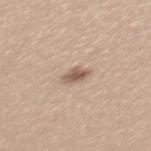Case summary:
– biopsy status — catalogued during a skin exam; not biopsied
– tile lighting — white-light illumination
– location — the mid back
– patient — female, about 25 years old
– image — ~15 mm crop, total-body skin-cancer survey